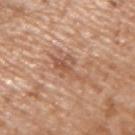Recorded during total-body skin imaging; not selected for excision or biopsy. The lesion's longest dimension is about 5 mm. A lesion tile, about 15 mm wide, cut from a 3D total-body photograph. A male subject roughly 65 years of age. On the right upper arm.